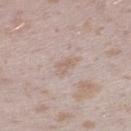Imaged during a routine full-body skin examination; the lesion was not biopsied and no histopathology is available.
From the right lower leg.
A region of skin cropped from a whole-body photographic capture, roughly 15 mm wide.
Captured under white-light illumination.
The lesion's longest dimension is about 2.5 mm.
A female subject aged 23 to 27.
Automated image analysis of the tile measured an area of roughly 3 mm² and a shape-asymmetry score of about 0.4 (0 = symmetric).